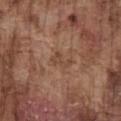The lesion was tiled from a total-body skin photograph and was not biopsied.
The subject is a male in their mid-70s.
Measured at roughly 3 mm in maximum diameter.
Imaged with white-light lighting.
A roughly 15 mm field-of-view crop from a total-body skin photograph.
The lesion is located on the chest.